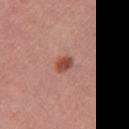The lesion was photographed on a routine skin check and not biopsied; there is no pathology result.
The lesion-visualizer software estimated a border-irregularity rating of about 1.5/10 and a within-lesion color-variation index near 3.5/10. And it measured a nevus-likeness score of about 95/100.
About 2.5 mm across.
A region of skin cropped from a whole-body photographic capture, roughly 15 mm wide.
The lesion is on the left upper arm.
Imaged with white-light lighting.
A female subject aged 23–27.